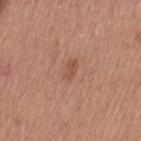workup — catalogued during a skin exam; not biopsied | subject — female, aged 38–42 | location — the leg | acquisition — ~15 mm tile from a whole-body skin photo.